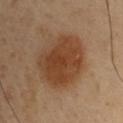Part of a total-body skin-imaging series; this lesion was reviewed on a skin check and was not flagged for biopsy. A male subject, roughly 55 years of age. From the chest. The total-body-photography lesion software estimated a classifier nevus-likeness of about 100/100 and a lesion-detection confidence of about 100/100. Captured under cross-polarized illumination. The lesion's longest dimension is about 6 mm. This image is a 15 mm lesion crop taken from a total-body photograph.